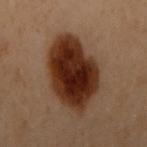This lesion was catalogued during total-body skin photography and was not selected for biopsy.
This is a cross-polarized tile.
A female subject, aged around 60.
A region of skin cropped from a whole-body photographic capture, roughly 15 mm wide.
From the back.
The recorded lesion diameter is about 7.5 mm.